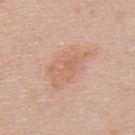No biopsy was performed on this lesion — it was imaged during a full skin examination and was not determined to be concerning. Located on the upper back. Automated image analysis of the tile measured an area of roughly 15 mm², an outline eccentricity of about 0.85 (0 = round, 1 = elongated), and a shape-asymmetry score of about 0.25 (0 = symmetric). And it measured a border-irregularity rating of about 3.5/10, internal color variation of about 3 on a 0–10 scale, and radial color variation of about 1. And it measured a nevus-likeness score of about 15/100. A male subject, aged 43–47. This image is a 15 mm lesion crop taken from a total-body photograph. Captured under white-light illumination. The recorded lesion diameter is about 6 mm.